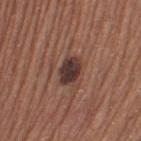Case summary:
- workup — imaged on a skin check; not biopsied
- lesion diameter — ~3.5 mm (longest diameter)
- patient — female, aged 63–67
- image — ~15 mm tile from a whole-body skin photo
- anatomic site — the left thigh
- lighting — white-light illumination